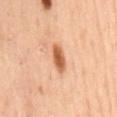Part of a total-body skin-imaging series; this lesion was reviewed on a skin check and was not flagged for biopsy. The lesion is on the mid back. Imaged with cross-polarized lighting. A male patient aged 53–57. The recorded lesion diameter is about 4 mm. A 15 mm close-up tile from a total-body photography series done for melanoma screening.